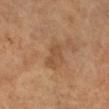Q: Is there a histopathology result?
A: catalogued during a skin exam; not biopsied
Q: Where on the body is the lesion?
A: the leg
Q: Automated lesion metrics?
A: a lesion area of about 3.5 mm² and a shape eccentricity near 0.85
Q: How large is the lesion?
A: ~3 mm (longest diameter)
Q: What kind of image is this?
A: ~15 mm tile from a whole-body skin photo
Q: Who is the patient?
A: female, aged 53 to 57
Q: How was the tile lit?
A: cross-polarized